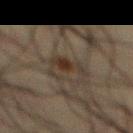subject — male, roughly 60 years of age | image source — ~15 mm tile from a whole-body skin photo | site — the chest | lighting — cross-polarized illumination | lesion diameter — ~4.5 mm (longest diameter).